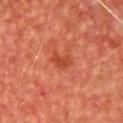Case summary:
– workup · no biopsy performed (imaged during a skin exam)
– location · the chest
– size · about 2.5 mm
– patient · male, approximately 65 years of age
– tile lighting · cross-polarized illumination
– image · ~15 mm tile from a whole-body skin photo